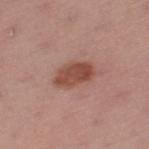Findings:
* follow-up · catalogued during a skin exam; not biopsied
* automated metrics · an area of roughly 9.5 mm², a shape eccentricity near 0.85, and a symmetry-axis asymmetry near 0.2; about 12 CIELAB-L* units darker than the surrounding skin; a classifier nevus-likeness of about 90/100
* image source · 15 mm crop, total-body photography
* diameter · about 4.5 mm
* site · the left thigh
* tile lighting · white-light illumination
* patient · female, roughly 55 years of age